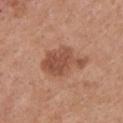Notes:
– workup — imaged on a skin check; not biopsied
– lesion size — ~5.5 mm (longest diameter)
– site — the chest
– image-analysis metrics — border irregularity of about 5 on a 0–10 scale, internal color variation of about 2.5 on a 0–10 scale, and peripheral color asymmetry of about 1; a nevus-likeness score of about 50/100 and a lesion-detection confidence of about 100/100
– image — ~15 mm tile from a whole-body skin photo
– subject — female, roughly 65 years of age